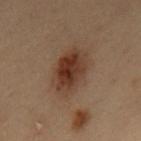Notes:
• notes: no biopsy performed (imaged during a skin exam)
• illumination: cross-polarized illumination
• TBP lesion metrics: a lesion area of about 15 mm², an outline eccentricity of about 0.85 (0 = round, 1 = elongated), and a symmetry-axis asymmetry near 0.2
• patient: male, in their mid-50s
• location: the mid back
• imaging modality: 15 mm crop, total-body photography